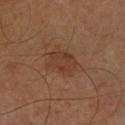No biopsy was performed on this lesion — it was imaged during a full skin examination and was not determined to be concerning. A male patient aged approximately 60. Automated tile analysis of the lesion measured a mean CIELAB color near L≈36 a*≈20 b*≈29, about 6 CIELAB-L* units darker than the surrounding skin, and a lesion-to-skin contrast of about 6 (normalized; higher = more distinct). A 15 mm crop from a total-body photograph taken for skin-cancer surveillance. Imaged with cross-polarized lighting. On the right forearm.